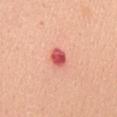size — ≈2.5 mm | location — the mid back | subject — female, aged 48 to 52 | image — ~15 mm crop, total-body skin-cancer survey.